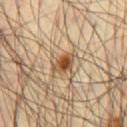The lesion was tiled from a total-body skin photograph and was not biopsied. The lesion is located on the chest. The patient is a male aged 63–67. A 15 mm close-up extracted from a 3D total-body photography capture. Approximately 3.5 mm at its widest. This is a cross-polarized tile.